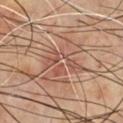Part of a total-body skin-imaging series; this lesion was reviewed on a skin check and was not flagged for biopsy. Automated tile analysis of the lesion measured an outline eccentricity of about 0.95 (0 = round, 1 = elongated) and a shape-asymmetry score of about 0.7 (0 = symmetric). A male subject, aged 68 to 72. A close-up tile cropped from a whole-body skin photograph, about 15 mm across. Imaged with cross-polarized lighting. About 5 mm across. The lesion is located on the front of the torso.